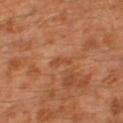Clinical impression: Recorded during total-body skin imaging; not selected for excision or biopsy. Acquisition and patient details: From the left thigh. A male subject, in their 30s. A roughly 15 mm field-of-view crop from a total-body skin photograph.